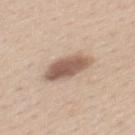{"biopsy_status": "not biopsied; imaged during a skin examination", "patient": {"sex": "male", "age_approx": 30}, "site": "mid back", "automated_metrics": {"border_irregularity_0_10": 2.5, "color_variation_0_10": 4.0, "nevus_likeness_0_100": 70, "lesion_detection_confidence_0_100": 100}, "lighting": "white-light", "lesion_size": {"long_diameter_mm_approx": 5.5}, "image": {"source": "total-body photography crop", "field_of_view_mm": 15}}